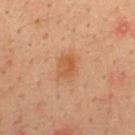The lesion was tiled from a total-body skin photograph and was not biopsied. The lesion is on the upper back. A close-up tile cropped from a whole-body skin photograph, about 15 mm across. A male subject about 35 years old.